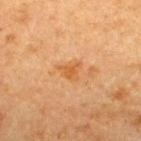Recorded during total-body skin imaging; not selected for excision or biopsy. On the upper back. A close-up tile cropped from a whole-body skin photograph, about 15 mm across. The lesion's longest dimension is about 2.5 mm. Automated tile analysis of the lesion measured border irregularity of about 4 on a 0–10 scale, a within-lesion color-variation index near 1/10, and a peripheral color-asymmetry measure near 0.5. And it measured an automated nevus-likeness rating near 0 out of 100. A male subject, aged around 50.